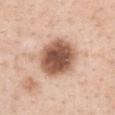Assessment:
Imaged during a routine full-body skin examination; the lesion was not biopsied and no histopathology is available.
Background:
From the chest. A region of skin cropped from a whole-body photographic capture, roughly 15 mm wide. Captured under white-light illumination. The recorded lesion diameter is about 5.5 mm. A female subject aged approximately 30.